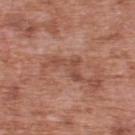notes: catalogued during a skin exam; not biopsied | patient: male, about 70 years old | lesion diameter: ~5.5 mm (longest diameter) | imaging modality: total-body-photography crop, ~15 mm field of view | automated lesion analysis: an area of roughly 6 mm² and a shape-asymmetry score of about 0.7 (0 = symmetric); border irregularity of about 10 on a 0–10 scale, internal color variation of about 0 on a 0–10 scale, and radial color variation of about 0; lesion-presence confidence of about 85/100 | site: the upper back | illumination: white-light.